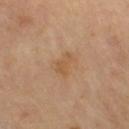{
  "biopsy_status": "not biopsied; imaged during a skin examination",
  "site": "left leg",
  "lesion_size": {
    "long_diameter_mm_approx": 3.0
  },
  "image": {
    "source": "total-body photography crop",
    "field_of_view_mm": 15
  },
  "patient": {
    "sex": "male",
    "age_approx": 65
  }
}